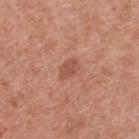Case summary:
- workup · imaged on a skin check; not biopsied
- body site · the upper back
- image · 15 mm crop, total-body photography
- patient · female, aged 33 to 37